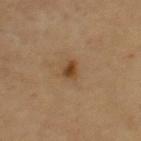Acquisition and patient details:
A region of skin cropped from a whole-body photographic capture, roughly 15 mm wide. The subject is a female aged 68–72. On the right upper arm.